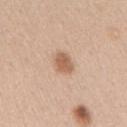Recorded during total-body skin imaging; not selected for excision or biopsy.
The tile uses white-light illumination.
Cropped from a whole-body photographic skin survey; the tile spans about 15 mm.
A female subject, aged 38 to 42.
The lesion is on the right upper arm.
About 2.5 mm across.